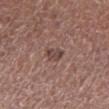| feature | finding |
|---|---|
| notes | total-body-photography surveillance lesion; no biopsy |
| image source | 15 mm crop, total-body photography |
| subject | male, about 75 years old |
| site | the left lower leg |